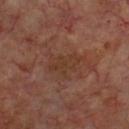Part of a total-body skin-imaging series; this lesion was reviewed on a skin check and was not flagged for biopsy. Cropped from a total-body skin-imaging series; the visible field is about 15 mm. On the front of the torso. Captured under cross-polarized illumination. A male subject about 70 years old.